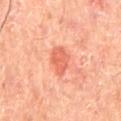Impression:
This lesion was catalogued during total-body skin photography and was not selected for biopsy.
Acquisition and patient details:
The lesion is on the back. This is a cross-polarized tile. Cropped from a total-body skin-imaging series; the visible field is about 15 mm. The lesion's longest dimension is about 3.5 mm. The lesion-visualizer software estimated a mean CIELAB color near L≈63 a*≈35 b*≈37 and a normalized lesion–skin contrast near 6.5. And it measured border irregularity of about 2.5 on a 0–10 scale, a color-variation rating of about 2/10, and a peripheral color-asymmetry measure near 1. The software also gave a nevus-likeness score of about 25/100 and lesion-presence confidence of about 100/100. A male patient, aged around 65.